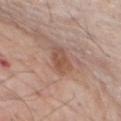Captured during whole-body skin photography for melanoma surveillance; the lesion was not biopsied. From the chest. A 15 mm close-up tile from a total-body photography series done for melanoma screening. A male patient approximately 80 years of age. Measured at roughly 4.5 mm in maximum diameter.